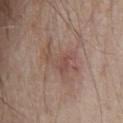Assessment:
Part of a total-body skin-imaging series; this lesion was reviewed on a skin check and was not flagged for biopsy.
Acquisition and patient details:
Approximately 5 mm at its widest. A male subject, aged around 80. Located on the chest. A 15 mm close-up tile from a total-body photography series done for melanoma screening. This is a white-light tile.